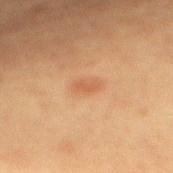biopsy status = catalogued during a skin exam; not biopsied